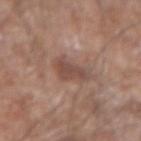This lesion was catalogued during total-body skin photography and was not selected for biopsy.
The lesion is located on the left forearm.
A male patient aged 58 to 62.
A region of skin cropped from a whole-body photographic capture, roughly 15 mm wide.
Approximately 3.5 mm at its widest.